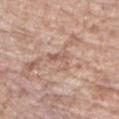<lesion>
<lighting>white-light</lighting>
<image>
  <source>total-body photography crop</source>
  <field_of_view_mm>15</field_of_view_mm>
</image>
<patient>
  <sex>female</sex>
  <age_approx>75</age_approx>
</patient>
<site>right upper arm</site>
<automated_metrics>
  <area_mm2_approx>3.5</area_mm2_approx>
  <eccentricity>0.7</eccentricity>
  <shape_asymmetry>0.65</shape_asymmetry>
  <cielab_L>58</cielab_L>
  <cielab_a>20</cielab_a>
  <cielab_b>27</cielab_b>
  <nevus_likeness_0_100>0</nevus_likeness_0_100>
  <lesion_detection_confidence_0_100>55</lesion_detection_confidence_0_100>
</automated_metrics>
</lesion>